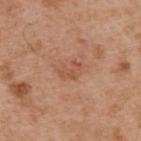workup: total-body-photography surveillance lesion; no biopsy
automated metrics: a mean CIELAB color near L≈53 a*≈24 b*≈32, a lesion–skin lightness drop of about 7, and a lesion-to-skin contrast of about 5 (normalized; higher = more distinct)
acquisition: ~15 mm tile from a whole-body skin photo
diameter: about 3 mm
lighting: white-light
subject: male, approximately 55 years of age
site: the upper back